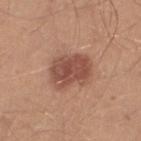Assessment:
Captured during whole-body skin photography for melanoma surveillance; the lesion was not biopsied.
Acquisition and patient details:
Cropped from a total-body skin-imaging series; the visible field is about 15 mm. A male patient, aged 28–32. The tile uses white-light illumination. From the left lower leg. The lesion-visualizer software estimated a mean CIELAB color near L≈50 a*≈23 b*≈28, a lesion–skin lightness drop of about 11, and a lesion-to-skin contrast of about 8 (normalized; higher = more distinct). And it measured a border-irregularity rating of about 2/10 and a color-variation rating of about 4/10. The lesion's longest dimension is about 5 mm.